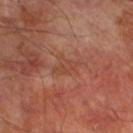The lesion was photographed on a routine skin check and not biopsied; there is no pathology result. An algorithmic analysis of the crop reported an area of roughly 4.5 mm², an eccentricity of roughly 0.8, and two-axis asymmetry of about 0.35. This image is a 15 mm lesion crop taken from a total-body photograph. A male patient aged approximately 70. Imaged with cross-polarized lighting. On the leg.